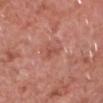Q: Lesion location?
A: the head or neck
Q: What lighting was used for the tile?
A: white-light
Q: What kind of image is this?
A: total-body-photography crop, ~15 mm field of view
Q: Automated lesion metrics?
A: a within-lesion color-variation index near 1/10 and a peripheral color-asymmetry measure near 0.5; an automated nevus-likeness rating near 5 out of 100 and lesion-presence confidence of about 100/100
Q: What is the lesion's diameter?
A: ≈2.5 mm
Q: Who is the patient?
A: male, about 70 years old